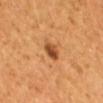Impression:
Part of a total-body skin-imaging series; this lesion was reviewed on a skin check and was not flagged for biopsy.
Image and clinical context:
Imaged with cross-polarized lighting. A region of skin cropped from a whole-body photographic capture, roughly 15 mm wide. The lesion is on the back. Approximately 3 mm at its widest. A male subject, aged 43–47.